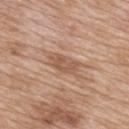Q: Was a biopsy performed?
A: imaged on a skin check; not biopsied
Q: What did automated image analysis measure?
A: a lesion area of about 6.5 mm²; a mean CIELAB color near L≈55 a*≈20 b*≈30, about 9 CIELAB-L* units darker than the surrounding skin, and a lesion-to-skin contrast of about 6.5 (normalized; higher = more distinct); border irregularity of about 2.5 on a 0–10 scale, internal color variation of about 2.5 on a 0–10 scale, and peripheral color asymmetry of about 1
Q: Where on the body is the lesion?
A: the upper back
Q: What are the patient's age and sex?
A: female, approximately 40 years of age
Q: What is the lesion's diameter?
A: ≈4 mm
Q: What is the imaging modality?
A: total-body-photography crop, ~15 mm field of view